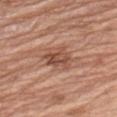Part of a total-body skin-imaging series; this lesion was reviewed on a skin check and was not flagged for biopsy. Cropped from a whole-body photographic skin survey; the tile spans about 15 mm. From the left upper arm. A female subject aged around 75. The total-body-photography lesion software estimated a footprint of about 8 mm². It also reported a lesion color around L≈50 a*≈22 b*≈29 in CIELAB, roughly 10 lightness units darker than nearby skin, and a normalized lesion–skin contrast near 7.5. The software also gave a border-irregularity rating of about 3.5/10, a within-lesion color-variation index near 5/10, and radial color variation of about 2. It also reported an automated nevus-likeness rating near 5 out of 100 and a lesion-detection confidence of about 100/100. Imaged with white-light lighting.